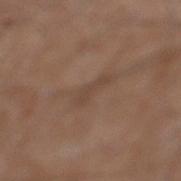biopsy_status: not biopsied; imaged during a skin examination